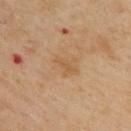{"biopsy_status": "not biopsied; imaged during a skin examination", "patient": {"sex": "male", "age_approx": 55}, "site": "upper back", "automated_metrics": {"area_mm2_approx": 2.5, "eccentricity": 0.9}, "lesion_size": {"long_diameter_mm_approx": 2.5}, "image": {"source": "total-body photography crop", "field_of_view_mm": 15}, "lighting": "cross-polarized"}